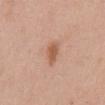Impression:
The lesion was tiled from a total-body skin photograph and was not biopsied.
Image and clinical context:
The lesion is located on the abdomen. Cropped from a total-body skin-imaging series; the visible field is about 15 mm. A female subject in their 50s.